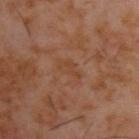Recorded during total-body skin imaging; not selected for excision or biopsy. This image is a 15 mm lesion crop taken from a total-body photograph. Automated image analysis of the tile measured a lesion area of about 4 mm², a shape eccentricity near 0.85, and a symmetry-axis asymmetry near 0.4. The analysis additionally found an average lesion color of about L≈40 a*≈20 b*≈29 (CIELAB) and a lesion–skin lightness drop of about 4. And it measured a border-irregularity index near 4.5/10, internal color variation of about 1.5 on a 0–10 scale, and radial color variation of about 0.5. On the upper back. Imaged with cross-polarized lighting. A male patient, aged 58 to 62. Longest diameter approximately 3 mm.